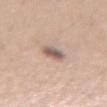Q: Was this lesion biopsied?
A: total-body-photography surveillance lesion; no biopsy
Q: Illumination type?
A: white-light illumination
Q: Patient demographics?
A: female, in their mid-20s
Q: What kind of image is this?
A: total-body-photography crop, ~15 mm field of view
Q: Lesion location?
A: the left forearm